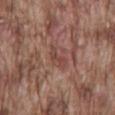A lesion tile, about 15 mm wide, cut from a 3D total-body photograph. The lesion is on the mid back. The patient is a male aged 73–77.